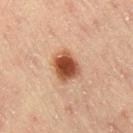{"biopsy_status": "not biopsied; imaged during a skin examination", "automated_metrics": {"border_irregularity_0_10": 1.5, "color_variation_0_10": 6.0, "peripheral_color_asymmetry": 1.5, "nevus_likeness_0_100": 100, "lesion_detection_confidence_0_100": 100}, "image": {"source": "total-body photography crop", "field_of_view_mm": 15}, "lesion_size": {"long_diameter_mm_approx": 3.5}, "site": "right thigh", "lighting": "cross-polarized", "patient": {"sex": "male", "age_approx": 45}}